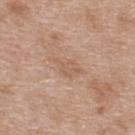Notes:
• workup · no biopsy performed (imaged during a skin exam)
• subject · female, aged around 40
• size · ~4 mm (longest diameter)
• location · the upper back
• image-analysis metrics · an area of roughly 5.5 mm², an eccentricity of roughly 0.85, and a shape-asymmetry score of about 0.45 (0 = symmetric); a lesion color around L≈59 a*≈19 b*≈31 in CIELAB and a normalized border contrast of about 4.5; a border-irregularity rating of about 5/10, a color-variation rating of about 1/10, and radial color variation of about 0.5; a classifier nevus-likeness of about 0/100 and a detector confidence of about 100 out of 100 that the crop contains a lesion
• illumination · white-light
• image source · total-body-photography crop, ~15 mm field of view